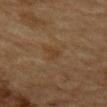Located on the mid back.
The patient is a male about 85 years old or older.
Approximately 2.5 mm at its widest.
Cropped from a whole-body photographic skin survey; the tile spans about 15 mm.
The tile uses cross-polarized illumination.